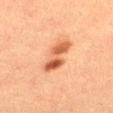Captured during whole-body skin photography for melanoma surveillance; the lesion was not biopsied. The tile uses cross-polarized illumination. The lesion is located on the right thigh. Cropped from a total-body skin-imaging series; the visible field is about 15 mm. The patient is a female aged 53–57. Automated tile analysis of the lesion measured a footprint of about 10 mm² and a symmetry-axis asymmetry near 0.25. Approximately 5 mm at its widest.